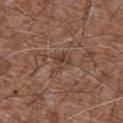Context:
A male patient aged approximately 75. Cropped from a whole-body photographic skin survey; the tile spans about 15 mm. Automated image analysis of the tile measured a lesion area of about 5 mm², an outline eccentricity of about 0.55 (0 = round, 1 = elongated), and a shape-asymmetry score of about 0.4 (0 = symmetric). The software also gave a border-irregularity index near 4.5/10, a color-variation rating of about 4.5/10, and a peripheral color-asymmetry measure near 1.5. It also reported an automated nevus-likeness rating near 0 out of 100 and a lesion-detection confidence of about 65/100. On the abdomen.